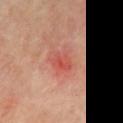{"biopsy_status": "not biopsied; imaged during a skin examination", "image": {"source": "total-body photography crop", "field_of_view_mm": 15}, "patient": {"sex": "male", "age_approx": 60}, "site": "chest", "automated_metrics": {"area_mm2_approx": 6.5, "eccentricity": 0.6, "shape_asymmetry": 0.25, "cielab_L": 52, "cielab_a": 31, "cielab_b": 30, "vs_skin_darker_L": 7.0, "vs_skin_contrast_norm": 5.5, "border_irregularity_0_10": 2.5, "color_variation_0_10": 5.5, "peripheral_color_asymmetry": 2.0}, "lighting": "cross-polarized", "lesion_size": {"long_diameter_mm_approx": 3.0}}